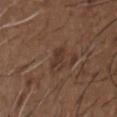Impression:
This lesion was catalogued during total-body skin photography and was not selected for biopsy.
Clinical summary:
Approximately 3 mm at its widest. A male subject, aged approximately 50. The lesion is on the front of the torso. Captured under white-light illumination. Cropped from a total-body skin-imaging series; the visible field is about 15 mm.